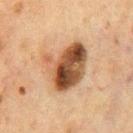Findings:
* lesion diameter: ~6 mm (longest diameter)
* body site: the chest
* lighting: cross-polarized illumination
* acquisition: ~15 mm crop, total-body skin-cancer survey
* subject: male, aged approximately 75